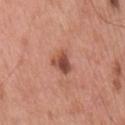Findings:
- image source · total-body-photography crop, ~15 mm field of view
- subject · male, aged 53 to 57
- lighting · white-light illumination
- anatomic site · the mid back
- automated metrics · an average lesion color of about L≈49 a*≈26 b*≈30 (CIELAB) and a lesion–skin lightness drop of about 13; a border-irregularity index near 3/10, a color-variation rating of about 5.5/10, and peripheral color asymmetry of about 2